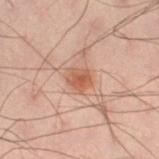| field | value |
|---|---|
| workup | total-body-photography surveillance lesion; no biopsy |
| image source | ~15 mm crop, total-body skin-cancer survey |
| lesion diameter | ~3 mm (longest diameter) |
| subject | male, aged 38 to 42 |
| tile lighting | cross-polarized illumination |
| location | the leg |